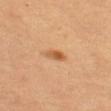Q: Was this lesion biopsied?
A: catalogued during a skin exam; not biopsied
Q: Automated lesion metrics?
A: a shape eccentricity near 0.85 and two-axis asymmetry of about 0.25; a border-irregularity rating of about 2/10 and a within-lesion color-variation index near 4/10; a nevus-likeness score of about 95/100 and a lesion-detection confidence of about 100/100
Q: How was this image acquired?
A: ~15 mm crop, total-body skin-cancer survey
Q: Where on the body is the lesion?
A: the left thigh
Q: What are the patient's age and sex?
A: female, aged around 50
Q: How large is the lesion?
A: ~2.5 mm (longest diameter)
Q: What lighting was used for the tile?
A: cross-polarized illumination